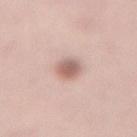{"biopsy_status": "not biopsied; imaged during a skin examination"}